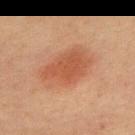<record>
  <biopsy_status>not biopsied; imaged during a skin examination</biopsy_status>
  <site>mid back</site>
  <patient>
    <sex>male</sex>
    <age_approx>60</age_approx>
  </patient>
  <image>
    <source>total-body photography crop</source>
    <field_of_view_mm>15</field_of_view_mm>
  </image>
  <lesion_size>
    <long_diameter_mm_approx>6.0</long_diameter_mm_approx>
  </lesion_size>
  <lighting>cross-polarized</lighting>
</record>